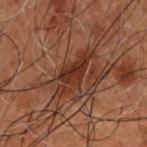follow-up=no biopsy performed (imaged during a skin exam) | imaging modality=~15 mm crop, total-body skin-cancer survey | size=~6 mm (longest diameter) | location=the upper back | lighting=cross-polarized illumination | patient=male, aged approximately 50.